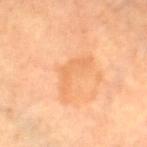This is a cross-polarized tile.
A female subject aged approximately 70.
Located on the leg.
The recorded lesion diameter is about 5.5 mm.
A close-up tile cropped from a whole-body skin photograph, about 15 mm across.